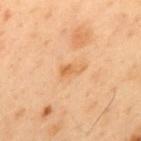Captured during whole-body skin photography for melanoma surveillance; the lesion was not biopsied. A 15 mm close-up extracted from a 3D total-body photography capture. Longest diameter approximately 3 mm. From the mid back. A male patient, aged 53–57.